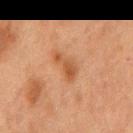Impression:
Part of a total-body skin-imaging series; this lesion was reviewed on a skin check and was not flagged for biopsy.
Clinical summary:
Measured at roughly 4.5 mm in maximum diameter. The tile uses cross-polarized illumination. A 15 mm crop from a total-body photograph taken for skin-cancer surveillance. From the mid back. A male subject, in their mid-70s. The total-body-photography lesion software estimated an outline eccentricity of about 0.9 (0 = round, 1 = elongated) and a symmetry-axis asymmetry near 0.45. And it measured a border-irregularity index near 5/10, internal color variation of about 3 on a 0–10 scale, and a peripheral color-asymmetry measure near 1. And it measured a classifier nevus-likeness of about 0/100 and a lesion-detection confidence of about 100/100.